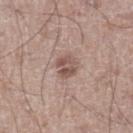follow-up: imaged on a skin check; not biopsied
imaging modality: total-body-photography crop, ~15 mm field of view
automated lesion analysis: an area of roughly 5 mm², an eccentricity of roughly 0.55, and a shape-asymmetry score of about 0.25 (0 = symmetric); a lesion color around L≈52 a*≈17 b*≈23 in CIELAB and a lesion-to-skin contrast of about 7.5 (normalized; higher = more distinct); a border-irregularity rating of about 2.5/10, a within-lesion color-variation index near 5/10, and radial color variation of about 2; a classifier nevus-likeness of about 30/100 and a lesion-detection confidence of about 100/100
subject: male, aged 18–22
size: about 2.5 mm
lighting: white-light
anatomic site: the right lower leg